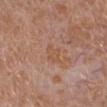Assessment: This lesion was catalogued during total-body skin photography and was not selected for biopsy. Acquisition and patient details: A close-up tile cropped from a whole-body skin photograph, about 15 mm across. The recorded lesion diameter is about 3 mm. Imaged with white-light lighting. The subject is a female aged around 55. Located on the right lower leg.